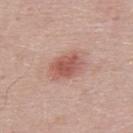Assessment: The lesion was photographed on a routine skin check and not biopsied; there is no pathology result. Acquisition and patient details: A male patient, aged 23 to 27. Located on the back. Cropped from a whole-body photographic skin survey; the tile spans about 15 mm.